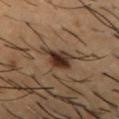Notes:
• follow-up: imaged on a skin check; not biopsied